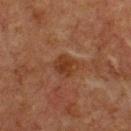{"biopsy_status": "not biopsied; imaged during a skin examination", "lighting": "cross-polarized", "patient": {"sex": "male", "age_approx": 75}, "lesion_size": {"long_diameter_mm_approx": 3.0}, "automated_metrics": {"eccentricity": 0.5, "shape_asymmetry": 0.2, "cielab_L": 29, "cielab_a": 19, "cielab_b": 27, "vs_skin_darker_L": 7.0, "vs_skin_contrast_norm": 7.5, "color_variation_0_10": 2.5, "peripheral_color_asymmetry": 1.0, "nevus_likeness_0_100": 55, "lesion_detection_confidence_0_100": 100}, "image": {"source": "total-body photography crop", "field_of_view_mm": 15}, "site": "chest"}